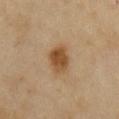follow-up: imaged on a skin check; not biopsied
subject: female, approximately 45 years of age
acquisition: ~15 mm crop, total-body skin-cancer survey
automated metrics: an eccentricity of roughly 0.65 and a symmetry-axis asymmetry near 0.2; border irregularity of about 1.5 on a 0–10 scale, a color-variation rating of about 3.5/10, and a peripheral color-asymmetry measure near 1; a detector confidence of about 100 out of 100 that the crop contains a lesion
site: the chest
lesion diameter: ~3.5 mm (longest diameter)